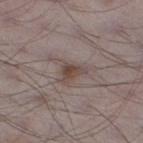Part of a total-body skin-imaging series; this lesion was reviewed on a skin check and was not flagged for biopsy. About 3 mm across. A roughly 15 mm field-of-view crop from a total-body skin photograph. Captured under white-light illumination. A male patient, aged around 70. The lesion is located on the left lower leg.